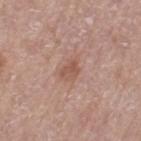follow-up: catalogued during a skin exam; not biopsied | size: ~2.5 mm (longest diameter) | image: total-body-photography crop, ~15 mm field of view | location: the left thigh | subject: female, approximately 75 years of age | illumination: white-light illumination.